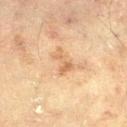Captured during whole-body skin photography for melanoma surveillance; the lesion was not biopsied. The lesion is on the leg. A male subject, approximately 70 years of age. A 15 mm close-up tile from a total-body photography series done for melanoma screening.